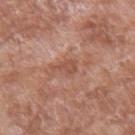Imaged during a routine full-body skin examination; the lesion was not biopsied and no histopathology is available.